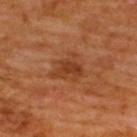workup = total-body-photography surveillance lesion; no biopsy | site = the upper back | diameter = about 4 mm | subject = male, aged approximately 65 | image = total-body-photography crop, ~15 mm field of view.